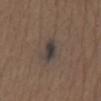Q: Was a biopsy performed?
A: catalogued during a skin exam; not biopsied
Q: How was the tile lit?
A: white-light
Q: Lesion location?
A: the chest
Q: What are the patient's age and sex?
A: male, about 75 years old
Q: How was this image acquired?
A: total-body-photography crop, ~15 mm field of view